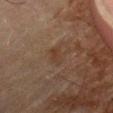notes: catalogued during a skin exam; not biopsied | image source: ~15 mm tile from a whole-body skin photo | location: the left thigh | automated lesion analysis: an area of roughly 3 mm², an outline eccentricity of about 0.85 (0 = round, 1 = elongated), and a symmetry-axis asymmetry near 0.35; a border-irregularity rating of about 3.5/10 and radial color variation of about 0 | subject: male, aged approximately 80 | lighting: cross-polarized illumination.